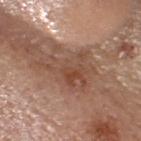This lesion was catalogued during total-body skin photography and was not selected for biopsy. The total-body-photography lesion software estimated a footprint of about 15 mm², an eccentricity of roughly 0.8, and two-axis asymmetry of about 0.55. It also reported a lesion color around L≈48 a*≈20 b*≈28 in CIELAB, about 9 CIELAB-L* units darker than the surrounding skin, and a lesion-to-skin contrast of about 7 (normalized; higher = more distinct). The software also gave internal color variation of about 5.5 on a 0–10 scale and peripheral color asymmetry of about 1.5. The analysis additionally found a detector confidence of about 75 out of 100 that the crop contains a lesion. The patient is a female roughly 65 years of age. Captured under white-light illumination. The lesion's longest dimension is about 6.5 mm. Located on the head or neck. A lesion tile, about 15 mm wide, cut from a 3D total-body photograph.